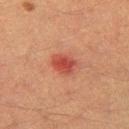Notes:
* notes: catalogued during a skin exam; not biopsied
* anatomic site: the left thigh
* diameter: about 3 mm
* tile lighting: cross-polarized
* patient: male, approximately 40 years of age
* acquisition: total-body-photography crop, ~15 mm field of view